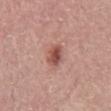<case>
<patient>
  <sex>male</sex>
  <age_approx>65</age_approx>
</patient>
<lesion_size>
  <long_diameter_mm_approx>3.0</long_diameter_mm_approx>
</lesion_size>
<site>back</site>
<image>
  <source>total-body photography crop</source>
  <field_of_view_mm>15</field_of_view_mm>
</image>
</case>